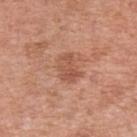Clinical impression:
This lesion was catalogued during total-body skin photography and was not selected for biopsy.
Background:
The tile uses white-light illumination. Located on the left forearm. A female patient, aged around 50. A roughly 15 mm field-of-view crop from a total-body skin photograph.